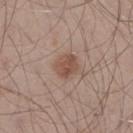Q: Was a biopsy performed?
A: no biopsy performed (imaged during a skin exam)
Q: What kind of image is this?
A: total-body-photography crop, ~15 mm field of view
Q: Automated lesion metrics?
A: a lesion area of about 6.5 mm², a shape eccentricity near 0.35, and a symmetry-axis asymmetry near 0.15
Q: Lesion size?
A: ≈3 mm
Q: Who is the patient?
A: male, aged approximately 35
Q: What is the anatomic site?
A: the leg